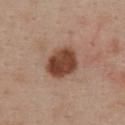Assessment:
The lesion was photographed on a routine skin check and not biopsied; there is no pathology result.
Background:
Automated image analysis of the tile measured a lesion area of about 12 mm² and a shape-asymmetry score of about 0.15 (0 = symmetric). The analysis additionally found an automated nevus-likeness rating near 95 out of 100. The subject is a female roughly 55 years of age. From the front of the torso. A 15 mm close-up tile from a total-body photography series done for melanoma screening.